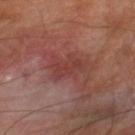<tbp_lesion>
  <image>
    <source>total-body photography crop</source>
    <field_of_view_mm>15</field_of_view_mm>
  </image>
  <site>right lower leg</site>
  <lighting>cross-polarized</lighting>
  <lesion_size>
    <long_diameter_mm_approx>4.5</long_diameter_mm_approx>
  </lesion_size>
  <automated_metrics>
    <area_mm2_approx>10.0</area_mm2_approx>
    <eccentricity>0.8</eccentricity>
    <shape_asymmetry>0.4</shape_asymmetry>
    <cielab_L>39</cielab_L>
    <cielab_a>25</cielab_a>
    <cielab_b>23</cielab_b>
    <vs_skin_contrast_norm>5.5</vs_skin_contrast_norm>
    <color_variation_0_10>2.5</color_variation_0_10>
    <peripheral_color_asymmetry>1.0</peripheral_color_asymmetry>
    <nevus_likeness_0_100>0</nevus_likeness_0_100>
    <lesion_detection_confidence_0_100>100</lesion_detection_confidence_0_100>
  </automated_metrics>
  <patient>
    <sex>male</sex>
    <age_approx>70</age_approx>
  </patient>
</tbp_lesion>